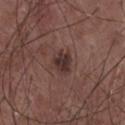biopsy status = total-body-photography surveillance lesion; no biopsy
lesion diameter = about 3 mm
location = the chest
subject = male, aged 58 to 62
image = ~15 mm tile from a whole-body skin photo